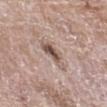Recorded during total-body skin imaging; not selected for excision or biopsy. A region of skin cropped from a whole-body photographic capture, roughly 15 mm wide. On the left thigh. Captured under white-light illumination. A female subject, aged 58–62.